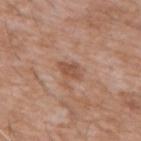Q: Was a biopsy performed?
A: catalogued during a skin exam; not biopsied
Q: What kind of image is this?
A: 15 mm crop, total-body photography
Q: How was the tile lit?
A: white-light illumination
Q: Who is the patient?
A: male, in their 60s
Q: Lesion location?
A: the chest